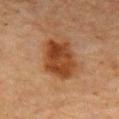{"biopsy_status": "not biopsied; imaged during a skin examination", "automated_metrics": {"area_mm2_approx": 16.0, "eccentricity": 0.8, "shape_asymmetry": 0.2, "border_irregularity_0_10": 2.5, "color_variation_0_10": 4.5, "peripheral_color_asymmetry": 1.5, "lesion_detection_confidence_0_100": 100}, "image": {"source": "total-body photography crop", "field_of_view_mm": 15}, "site": "front of the torso", "lighting": "cross-polarized", "patient": {"sex": "male", "age_approx": 80}}